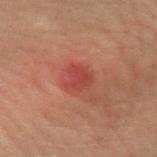A roughly 15 mm field-of-view crop from a total-body skin photograph.
The lesion is located on the left forearm.
The patient is a male aged 53 to 57.